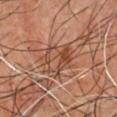Assessment:
No biopsy was performed on this lesion — it was imaged during a full skin examination and was not determined to be concerning.
Image and clinical context:
On the chest. A male patient in their mid- to late 60s. This is a cross-polarized tile. Automated image analysis of the tile measured a footprint of about 11 mm², a shape eccentricity near 0.55, and two-axis asymmetry of about 0.25. And it measured an average lesion color of about L≈48 a*≈22 b*≈31 (CIELAB) and roughly 8 lightness units darker than nearby skin. And it measured a detector confidence of about 70 out of 100 that the crop contains a lesion. A 15 mm close-up extracted from a 3D total-body photography capture.